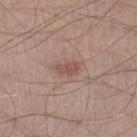Impression:
This lesion was catalogued during total-body skin photography and was not selected for biopsy.
Clinical summary:
Located on the right lower leg. The lesion's longest dimension is about 2.5 mm. The subject is a male in their mid- to late 50s. A close-up tile cropped from a whole-body skin photograph, about 15 mm across. An algorithmic analysis of the crop reported a lesion area of about 3.5 mm². The analysis additionally found internal color variation of about 1 on a 0–10 scale and peripheral color asymmetry of about 0.5.